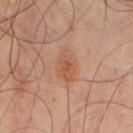Background: A male subject approximately 50 years of age. Approximately 4 mm at its widest. On the abdomen. A region of skin cropped from a whole-body photographic capture, roughly 15 mm wide. Imaged with cross-polarized lighting.